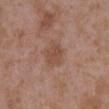Q: Is there a histopathology result?
A: imaged on a skin check; not biopsied